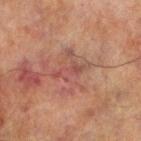follow-up = total-body-photography surveillance lesion; no biopsy | subject = male, approximately 70 years of age | acquisition = ~15 mm crop, total-body skin-cancer survey | site = the left lower leg.